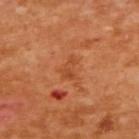{
  "biopsy_status": "not biopsied; imaged during a skin examination",
  "site": "upper back",
  "lighting": "cross-polarized",
  "automated_metrics": {
    "border_irregularity_0_10": 6.0,
    "color_variation_0_10": 1.5,
    "peripheral_color_asymmetry": 0.5
  },
  "patient": {
    "sex": "female",
    "age_approx": 55
  },
  "image": {
    "source": "total-body photography crop",
    "field_of_view_mm": 15
  }
}